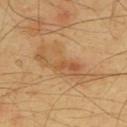Clinical impression: No biopsy was performed on this lesion — it was imaged during a full skin examination and was not determined to be concerning. Context: About 9 mm across. The tile uses cross-polarized illumination. Automated image analysis of the tile measured a lesion area of about 20 mm², an outline eccentricity of about 0.95 (0 = round, 1 = elongated), and a shape-asymmetry score of about 0.35 (0 = symmetric). The software also gave a lesion color around L≈57 a*≈19 b*≈39 in CIELAB, about 8 CIELAB-L* units darker than the surrounding skin, and a lesion-to-skin contrast of about 5.5 (normalized; higher = more distinct). A 15 mm crop from a total-body photograph taken for skin-cancer surveillance. On the upper back. A male patient, in their mid-60s.